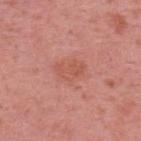<case>
  <biopsy_status>not biopsied; imaged during a skin examination</biopsy_status>
  <lesion_size>
    <long_diameter_mm_approx>3.5</long_diameter_mm_approx>
  </lesion_size>
  <patient>
    <sex>female</sex>
    <age_approx>40</age_approx>
  </patient>
  <image>
    <source>total-body photography crop</source>
    <field_of_view_mm>15</field_of_view_mm>
  </image>
  <lighting>white-light</lighting>
  <site>upper back</site>
  <automated_metrics>
    <area_mm2_approx>6.5</area_mm2_approx>
    <eccentricity>0.7</eccentricity>
    <shape_asymmetry>0.3</shape_asymmetry>
    <vs_skin_contrast_norm>5.5</vs_skin_contrast_norm>
    <nevus_likeness_0_100>5</nevus_likeness_0_100>
    <lesion_detection_confidence_0_100>100</lesion_detection_confidence_0_100>
  </automated_metrics>
</case>